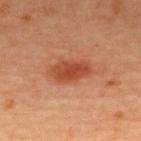Impression:
Captured during whole-body skin photography for melanoma surveillance; the lesion was not biopsied.
Context:
A female patient aged 43–47. A roughly 15 mm field-of-view crop from a total-body skin photograph. This is a cross-polarized tile. The lesion is located on the upper back. About 5 mm across.